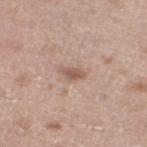Imaged during a routine full-body skin examination; the lesion was not biopsied and no histopathology is available. On the left lower leg. Cropped from a whole-body photographic skin survey; the tile spans about 15 mm. The total-body-photography lesion software estimated a footprint of about 3 mm² and a shape eccentricity near 0.85. A male subject about 65 years old.